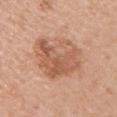The lesion was photographed on a routine skin check and not biopsied; there is no pathology result.
Captured under white-light illumination.
A 15 mm crop from a total-body photograph taken for skin-cancer surveillance.
A female subject, about 55 years old.
The total-body-photography lesion software estimated a footprint of about 22 mm², a shape eccentricity near 0.65, and two-axis asymmetry of about 0.25. The software also gave a classifier nevus-likeness of about 15/100 and a lesion-detection confidence of about 100/100.
On the left upper arm.